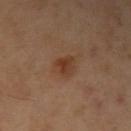biopsy status: total-body-photography surveillance lesion; no biopsy | automated lesion analysis: an outline eccentricity of about 0.6 (0 = round, 1 = elongated) and two-axis asymmetry of about 0.35; a mean CIELAB color near L≈37 a*≈19 b*≈30; a within-lesion color-variation index near 4.5/10 and radial color variation of about 1.5; a classifier nevus-likeness of about 90/100 and a lesion-detection confidence of about 100/100 | subject: male, in their 50s | site: the left arm | image source: ~15 mm crop, total-body skin-cancer survey | lesion diameter: ~3 mm (longest diameter) | tile lighting: cross-polarized.